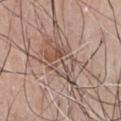follow-up — imaged on a skin check; not biopsied | site — the chest | image-analysis metrics — a mean CIELAB color near L≈52 a*≈17 b*≈25, about 9 CIELAB-L* units darker than the surrounding skin, and a normalized border contrast of about 6.5; a border-irregularity rating of about 5.5/10; an automated nevus-likeness rating near 0 out of 100 and lesion-presence confidence of about 70/100 | imaging modality — total-body-photography crop, ~15 mm field of view | subject — male, aged 48–52 | lighting — white-light illumination | lesion size — ≈7 mm.